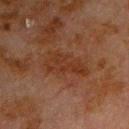Clinical impression:
Captured during whole-body skin photography for melanoma surveillance; the lesion was not biopsied.
Background:
Captured under cross-polarized illumination. The lesion is on the front of the torso. A male subject aged approximately 80. A 15 mm close-up extracted from a 3D total-body photography capture.